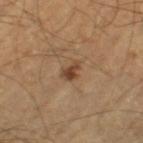Context:
The patient is a male approximately 55 years of age. Captured under cross-polarized illumination. A roughly 15 mm field-of-view crop from a total-body skin photograph. Located on the left thigh. About 2.5 mm across.